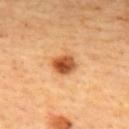Q: Is there a histopathology result?
A: total-body-photography surveillance lesion; no biopsy